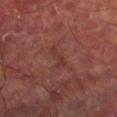Q: Is there a histopathology result?
A: total-body-photography surveillance lesion; no biopsy
Q: How was the tile lit?
A: cross-polarized
Q: What is the anatomic site?
A: the right lower leg
Q: Patient demographics?
A: male, roughly 60 years of age
Q: How was this image acquired?
A: ~15 mm crop, total-body skin-cancer survey
Q: Automated lesion metrics?
A: an automated nevus-likeness rating near 0 out of 100